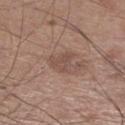The lesion was photographed on a routine skin check and not biopsied; there is no pathology result. The lesion is located on the right lower leg. A 15 mm close-up extracted from a 3D total-body photography capture. The patient is a male aged around 60. Captured under white-light illumination. The total-body-photography lesion software estimated a mean CIELAB color near L≈49 a*≈18 b*≈24 and about 7 CIELAB-L* units darker than the surrounding skin. The analysis additionally found a border-irregularity rating of about 4.5/10, a color-variation rating of about 1.5/10, and radial color variation of about 0.5. It also reported an automated nevus-likeness rating near 0 out of 100 and a lesion-detection confidence of about 100/100. Longest diameter approximately 3 mm.